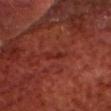Assessment:
This lesion was catalogued during total-body skin photography and was not selected for biopsy.
Context:
Approximately 3 mm at its widest. The tile uses cross-polarized illumination. A 15 mm crop from a total-body photograph taken for skin-cancer surveillance. Automated image analysis of the tile measured a lesion area of about 2.5 mm², a shape eccentricity near 0.95, and two-axis asymmetry of about 0.5. The lesion is located on the head or neck. A male patient, approximately 70 years of age.